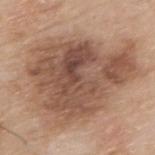A male subject, approximately 80 years of age.
On the arm.
The lesion-visualizer software estimated a normalized lesion–skin contrast near 9. It also reported a border-irregularity rating of about 4.5/10. The software also gave an automated nevus-likeness rating near 35 out of 100 and a lesion-detection confidence of about 100/100.
Imaged with white-light lighting.
Approximately 11.5 mm at its widest.
A region of skin cropped from a whole-body photographic capture, roughly 15 mm wide.
Histopathology of the biopsied lesion showed a melanoma in situ — a skin cancer.